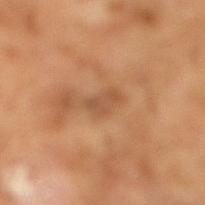  biopsy_status: not biopsied; imaged during a skin examination
  patient:
    sex: male
    age_approx: 65
  image:
    source: total-body photography crop
    field_of_view_mm: 15